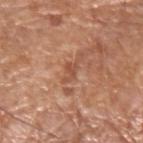biopsy status: imaged on a skin check; not biopsied | body site: the left upper arm | patient: male, aged 73–77 | lighting: white-light | acquisition: ~15 mm crop, total-body skin-cancer survey.